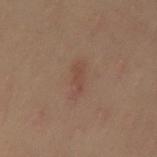{"biopsy_status": "not biopsied; imaged during a skin examination", "site": "right leg", "image": {"source": "total-body photography crop", "field_of_view_mm": 15}, "patient": {"sex": "female", "age_approx": 60}}